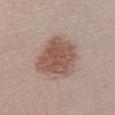{
  "biopsy_status": "not biopsied; imaged during a skin examination",
  "site": "leg",
  "image": {
    "source": "total-body photography crop",
    "field_of_view_mm": 15
  },
  "patient": {
    "sex": "male",
    "age_approx": 50
  }
}